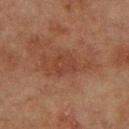Clinical impression: Imaged during a routine full-body skin examination; the lesion was not biopsied and no histopathology is available. Acquisition and patient details: Cropped from a total-body skin-imaging series; the visible field is about 15 mm. Measured at roughly 3 mm in maximum diameter. The lesion is on the left forearm. The patient is a female approximately 80 years of age. Captured under cross-polarized illumination.